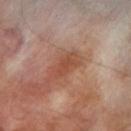The lesion was tiled from a total-body skin photograph and was not biopsied. The tile uses cross-polarized illumination. An algorithmic analysis of the crop reported an eccentricity of roughly 0.85 and a symmetry-axis asymmetry near 0.3. And it measured a peripheral color-asymmetry measure near 0.5. Approximately 3 mm at its widest. Located on the left thigh. A close-up tile cropped from a whole-body skin photograph, about 15 mm across. A male patient aged 68–72.